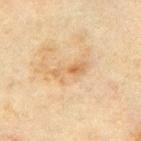<lesion>
<biopsy_status>not biopsied; imaged during a skin examination</biopsy_status>
<lesion_size>
  <long_diameter_mm_approx>4.0</long_diameter_mm_approx>
</lesion_size>
<automated_metrics>
  <eccentricity>0.9</eccentricity>
  <cielab_L>54</cielab_L>
  <cielab_a>15</cielab_a>
  <cielab_b>35</cielab_b>
  <vs_skin_darker_L>7.0</vs_skin_darker_L>
  <vs_skin_contrast_norm>5.5</vs_skin_contrast_norm>
</automated_metrics>
<image>
  <source>total-body photography crop</source>
  <field_of_view_mm>15</field_of_view_mm>
</image>
<site>front of the torso</site>
<patient>
  <sex>male</sex>
  <age_approx>70</age_approx>
</patient>
<lighting>cross-polarized</lighting>
</lesion>